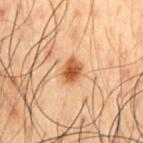Clinical impression: Recorded during total-body skin imaging; not selected for excision or biopsy. Background: A male patient approximately 50 years of age. Imaged with cross-polarized lighting. A roughly 15 mm field-of-view crop from a total-body skin photograph. The lesion is located on the chest. Automated image analysis of the tile measured an average lesion color of about L≈43 a*≈20 b*≈33 (CIELAB), roughly 11 lightness units darker than nearby skin, and a lesion-to-skin contrast of about 9.5 (normalized; higher = more distinct).